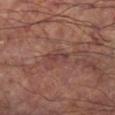Recorded during total-body skin imaging; not selected for excision or biopsy. From the leg. A male patient aged around 60. Cropped from a whole-body photographic skin survey; the tile spans about 15 mm. Captured under cross-polarized illumination. Automated image analysis of the tile measured a footprint of about 3.5 mm², a shape eccentricity near 0.9, and two-axis asymmetry of about 0.35. The analysis additionally found a mean CIELAB color near L≈39 a*≈22 b*≈21, roughly 7 lightness units darker than nearby skin, and a lesion-to-skin contrast of about 6 (normalized; higher = more distinct).